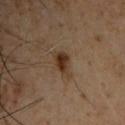No biopsy was performed on this lesion — it was imaged during a full skin examination and was not determined to be concerning. The patient is a male approximately 50 years of age. A 15 mm close-up extracted from a 3D total-body photography capture. The lesion is located on the chest.